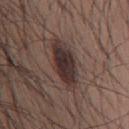biopsy status = total-body-photography surveillance lesion; no biopsy | image-analysis metrics = a lesion area of about 13 mm², a shape eccentricity near 0.9, and a symmetry-axis asymmetry near 0.2; a classifier nevus-likeness of about 85/100 and a detector confidence of about 100 out of 100 that the crop contains a lesion | lesion size = ≈6 mm | patient = male, aged around 35 | image source = 15 mm crop, total-body photography | body site = the mid back | tile lighting = white-light illumination.